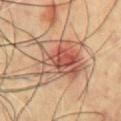biopsy_status: not biopsied; imaged during a skin examination
patient:
  sex: male
  age_approx: 70
image:
  source: total-body photography crop
  field_of_view_mm: 15
site: chest
lesion_size:
  long_diameter_mm_approx: 6.0
lighting: cross-polarized